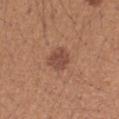| field | value |
|---|---|
| follow-up | catalogued during a skin exam; not biopsied |
| site | the right forearm |
| automated lesion analysis | an area of roughly 6.5 mm², a shape eccentricity near 0.65, and a shape-asymmetry score of about 0.25 (0 = symmetric); roughly 9 lightness units darker than nearby skin and a normalized border contrast of about 7.5; a color-variation rating of about 2/10 and peripheral color asymmetry of about 0.5; a classifier nevus-likeness of about 55/100 and a lesion-detection confidence of about 100/100 |
| imaging modality | ~15 mm crop, total-body skin-cancer survey |
| lesion diameter | ≈3 mm |
| subject | female, roughly 40 years of age |